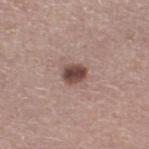<record>
<biopsy_status>not biopsied; imaged during a skin examination</biopsy_status>
<image>
  <source>total-body photography crop</source>
  <field_of_view_mm>15</field_of_view_mm>
</image>
<site>left lower leg</site>
<patient>
  <sex>male</sex>
  <age_approx>65</age_approx>
</patient>
</record>